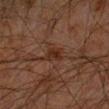No biopsy was performed on this lesion — it was imaged during a full skin examination and was not determined to be concerning. A male patient in their mid-60s. A 15 mm close-up tile from a total-body photography series done for melanoma screening. The lesion's longest dimension is about 2.5 mm. The lesion is located on the left forearm. The tile uses cross-polarized illumination.